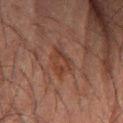Assessment:
The lesion was tiled from a total-body skin photograph and was not biopsied.
Acquisition and patient details:
Imaged with cross-polarized lighting. The lesion is located on the right forearm. A region of skin cropped from a whole-body photographic capture, roughly 15 mm wide. A male patient, aged around 70. The lesion's longest dimension is about 3.5 mm.